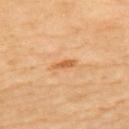Q: Is there a histopathology result?
A: total-body-photography surveillance lesion; no biopsy
Q: Automated lesion metrics?
A: a lesion area of about 3 mm², a shape eccentricity near 0.9, and two-axis asymmetry of about 0.35; a mean CIELAB color near L≈64 a*≈25 b*≈46 and a lesion–skin lightness drop of about 10; a classifier nevus-likeness of about 0/100 and a detector confidence of about 100 out of 100 that the crop contains a lesion
Q: How was this image acquired?
A: total-body-photography crop, ~15 mm field of view
Q: How large is the lesion?
A: about 3 mm
Q: What lighting was used for the tile?
A: cross-polarized illumination
Q: Where on the body is the lesion?
A: the upper back
Q: Patient demographics?
A: female, approximately 70 years of age